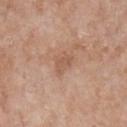workup: no biopsy performed (imaged during a skin exam)